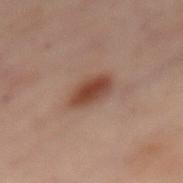Recorded during total-body skin imaging; not selected for excision or biopsy.
The lesion is on the back.
The subject is a female aged approximately 55.
Longest diameter approximately 4 mm.
A close-up tile cropped from a whole-body skin photograph, about 15 mm across.